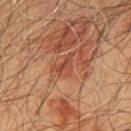The tile uses cross-polarized illumination. The lesion is on the chest. A male patient aged around 60. Measured at roughly 3 mm in maximum diameter. A 15 mm crop from a total-body photograph taken for skin-cancer surveillance.